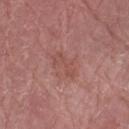<case>
  <biopsy_status>not biopsied; imaged during a skin examination</biopsy_status>
  <automated_metrics>
    <area_mm2_approx>4.5</area_mm2_approx>
    <eccentricity>0.9</eccentricity>
    <shape_asymmetry>0.4</shape_asymmetry>
  </automated_metrics>
  <image>
    <source>total-body photography crop</source>
    <field_of_view_mm>15</field_of_view_mm>
  </image>
  <site>left forearm</site>
  <lesion_size>
    <long_diameter_mm_approx>3.5</long_diameter_mm_approx>
  </lesion_size>
  <patient>
    <sex>male</sex>
    <age_approx>70</age_approx>
  </patient>
  <lighting>white-light</lighting>
</case>